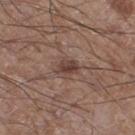The tile uses white-light illumination. The recorded lesion diameter is about 3 mm. The lesion is on the right thigh. Cropped from a total-body skin-imaging series; the visible field is about 15 mm. The subject is a male approximately 60 years of age.